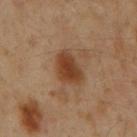The lesion is on the mid back. Cropped from a total-body skin-imaging series; the visible field is about 15 mm. The lesion-visualizer software estimated an area of roughly 9 mm², a shape eccentricity near 0.8, and two-axis asymmetry of about 0.25. The software also gave a lesion color around L≈37 a*≈19 b*≈30 in CIELAB, a lesion–skin lightness drop of about 10, and a lesion-to-skin contrast of about 9.5 (normalized; higher = more distinct). Approximately 4.5 mm at its widest. A male subject about 60 years old.